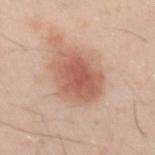<case>
<patient>
  <sex>male</sex>
  <age_approx>30</age_approx>
</patient>
<site>left upper arm</site>
<lesion_size>
  <long_diameter_mm_approx>6.0</long_diameter_mm_approx>
</lesion_size>
<automated_metrics>
  <eccentricity>0.7</eccentricity>
  <shape_asymmetry>0.15</shape_asymmetry>
  <border_irregularity_0_10>2.0</border_irregularity_0_10>
  <color_variation_0_10>4.0</color_variation_0_10>
  <peripheral_color_asymmetry>1.5</peripheral_color_asymmetry>
</automated_metrics>
<lighting>white-light</lighting>
<image>
  <source>total-body photography crop</source>
  <field_of_view_mm>15</field_of_view_mm>
</image>
</case>